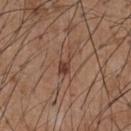Assessment: Part of a total-body skin-imaging series; this lesion was reviewed on a skin check and was not flagged for biopsy. Clinical summary: On the chest. Imaged with white-light lighting. The patient is a male approximately 55 years of age. Cropped from a whole-body photographic skin survey; the tile spans about 15 mm.